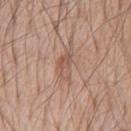biopsy status: no biopsy performed (imaged during a skin exam); acquisition: ~15 mm crop, total-body skin-cancer survey; patient: male, aged 53–57; diameter: ≈4 mm; anatomic site: the front of the torso; TBP lesion metrics: an automated nevus-likeness rating near 0 out of 100.